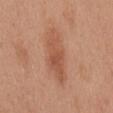No biopsy was performed on this lesion — it was imaged during a full skin examination and was not determined to be concerning.